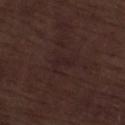| key | value |
|---|---|
| biopsy status | no biopsy performed (imaged during a skin exam) |
| illumination | white-light |
| size | ~2.5 mm (longest diameter) |
| image | 15 mm crop, total-body photography |
| patient | male, roughly 70 years of age |
| site | the leg |